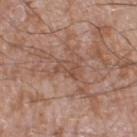<tbp_lesion>
  <biopsy_status>not biopsied; imaged during a skin examination</biopsy_status>
  <patient>
    <sex>male</sex>
    <age_approx>60</age_approx>
  </patient>
  <site>leg</site>
  <automated_metrics>
    <area_mm2_approx>4.0</area_mm2_approx>
    <eccentricity>0.8</eccentricity>
    <shape_asymmetry>0.25</shape_asymmetry>
    <cielab_L>49</cielab_L>
    <cielab_a>20</cielab_a>
    <cielab_b>28</cielab_b>
    <vs_skin_darker_L>7.0</vs_skin_darker_L>
    <vs_skin_contrast_norm>5.0</vs_skin_contrast_norm>
    <border_irregularity_0_10>2.5</border_irregularity_0_10>
    <color_variation_0_10>3.0</color_variation_0_10>
    <peripheral_color_asymmetry>1.0</peripheral_color_asymmetry>
    <nevus_likeness_0_100>0</nevus_likeness_0_100>
  </automated_metrics>
  <image>
    <source>total-body photography crop</source>
    <field_of_view_mm>15</field_of_view_mm>
  </image>
  <lighting>white-light</lighting>
  <lesion_size>
    <long_diameter_mm_approx>2.5</long_diameter_mm_approx>
  </lesion_size>
</tbp_lesion>